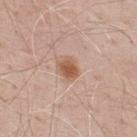Imaged during a routine full-body skin examination; the lesion was not biopsied and no histopathology is available. Located on the upper back. The total-body-photography lesion software estimated a footprint of about 5 mm². It also reported a mean CIELAB color near L≈56 a*≈21 b*≈32 and roughly 11 lightness units darker than nearby skin. It also reported border irregularity of about 1.5 on a 0–10 scale, a color-variation rating of about 3/10, and a peripheral color-asymmetry measure near 1. The software also gave an automated nevus-likeness rating near 95 out of 100 and lesion-presence confidence of about 100/100. Captured under white-light illumination. The patient is a male approximately 70 years of age. The recorded lesion diameter is about 2.5 mm. A close-up tile cropped from a whole-body skin photograph, about 15 mm across.